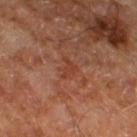{"biopsy_status": "not biopsied; imaged during a skin examination", "image": {"source": "total-body photography crop", "field_of_view_mm": 15}, "lighting": "cross-polarized", "site": "right thigh", "lesion_size": {"long_diameter_mm_approx": 2.5}, "patient": {"age_approx": 65}, "automated_metrics": {"area_mm2_approx": 3.0, "cielab_L": 40, "cielab_a": 26, "cielab_b": 31, "vs_skin_darker_L": 6.0, "vs_skin_contrast_norm": 5.5, "border_irregularity_0_10": 5.0, "color_variation_0_10": 1.0, "peripheral_color_asymmetry": 0.5}}